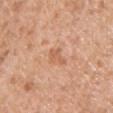Case summary:
• workup — total-body-photography surveillance lesion; no biopsy
• lighting — white-light illumination
• body site — the chest
• automated metrics — a mean CIELAB color near L≈61 a*≈24 b*≈35 and roughly 8 lightness units darker than nearby skin
• lesion diameter — about 3 mm
• subject — male, approximately 60 years of age
• imaging modality — ~15 mm tile from a whole-body skin photo